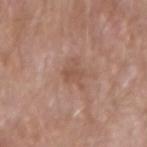{"biopsy_status": "not biopsied; imaged during a skin examination", "image": {"source": "total-body photography crop", "field_of_view_mm": 15}, "lighting": "white-light", "patient": {"sex": "male", "age_approx": 65}, "lesion_size": {"long_diameter_mm_approx": 2.5}, "site": "left forearm"}